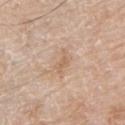The tile uses white-light illumination.
On the left upper arm.
A male patient, in their 80s.
The total-body-photography lesion software estimated a lesion area of about 3 mm², an eccentricity of roughly 0.9, and a shape-asymmetry score of about 0.5 (0 = symmetric). And it measured about 7 CIELAB-L* units darker than the surrounding skin. It also reported a border-irregularity rating of about 5/10 and a color-variation rating of about 0/10. The software also gave a lesion-detection confidence of about 100/100.
This image is a 15 mm lesion crop taken from a total-body photograph.